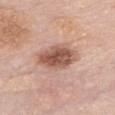Clinical impression:
Recorded during total-body skin imaging; not selected for excision or biopsy.
Background:
Imaged with white-light lighting. A female subject, aged 58–62. The lesion is on the front of the torso. The recorded lesion diameter is about 5 mm. This image is a 15 mm lesion crop taken from a total-body photograph. Automated image analysis of the tile measured a footprint of about 13 mm². And it measured a lesion color around L≈55 a*≈22 b*≈27 in CIELAB and a normalized lesion–skin contrast near 9.5. And it measured a nevus-likeness score of about 80/100 and lesion-presence confidence of about 100/100.